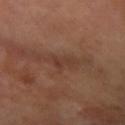The lesion was photographed on a routine skin check and not biopsied; there is no pathology result. Imaged with cross-polarized lighting. The lesion's longest dimension is about 2.5 mm. From the right forearm. Automated tile analysis of the lesion measured a lesion area of about 2.5 mm², an outline eccentricity of about 0.9 (0 = round, 1 = elongated), and a shape-asymmetry score of about 0.45 (0 = symmetric). The software also gave a lesion–skin lightness drop of about 6. The analysis additionally found a classifier nevus-likeness of about 0/100 and a detector confidence of about 90 out of 100 that the crop contains a lesion. A male patient roughly 70 years of age. Cropped from a total-body skin-imaging series; the visible field is about 15 mm.